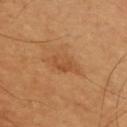Case summary:
* notes: total-body-photography surveillance lesion; no biopsy
* image-analysis metrics: a lesion color around L≈49 a*≈22 b*≈37 in CIELAB, a lesion–skin lightness drop of about 7, and a normalized border contrast of about 5; border irregularity of about 2.5 on a 0–10 scale, internal color variation of about 2.5 on a 0–10 scale, and a peripheral color-asymmetry measure near 1
* patient: male, aged 58 to 62
* illumination: cross-polarized illumination
* anatomic site: the chest
* imaging modality: total-body-photography crop, ~15 mm field of view
* diameter: ≈4 mm